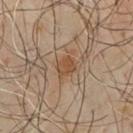notes = catalogued during a skin exam; not biopsied
diameter = ~2.5 mm (longest diameter)
automated lesion analysis = a mean CIELAB color near L≈46 a*≈18 b*≈31 and a normalized border contrast of about 7; a border-irregularity rating of about 2/10, a color-variation rating of about 2.5/10, and peripheral color asymmetry of about 1
site = the chest
image source = total-body-photography crop, ~15 mm field of view
patient = male, approximately 65 years of age
lighting = cross-polarized